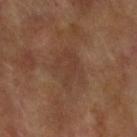On the left forearm.
The patient is a female aged approximately 75.
Cropped from a whole-body photographic skin survey; the tile spans about 15 mm.
Longest diameter approximately 5 mm.
Automated tile analysis of the lesion measured a lesion color around L≈39 a*≈18 b*≈27 in CIELAB, roughly 5 lightness units darker than nearby skin, and a lesion-to-skin contrast of about 5 (normalized; higher = more distinct). The software also gave a color-variation rating of about 2.5/10 and peripheral color asymmetry of about 1.
Imaged with cross-polarized lighting.